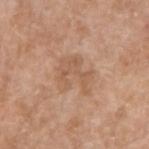Q: Was this lesion biopsied?
A: imaged on a skin check; not biopsied